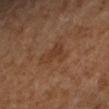No biopsy was performed on this lesion — it was imaged during a full skin examination and was not determined to be concerning. Cropped from a whole-body photographic skin survey; the tile spans about 15 mm. The subject is a female roughly 65 years of age. Automated image analysis of the tile measured a lesion area of about 6.5 mm², an outline eccentricity of about 0.7 (0 = round, 1 = elongated), and a shape-asymmetry score of about 0.55 (0 = symmetric). And it measured border irregularity of about 5.5 on a 0–10 scale, a color-variation rating of about 2/10, and a peripheral color-asymmetry measure near 0.5. And it measured a nevus-likeness score of about 0/100. From the right forearm. Captured under cross-polarized illumination.